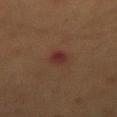Part of a total-body skin-imaging series; this lesion was reviewed on a skin check and was not flagged for biopsy. A 15 mm close-up extracted from a 3D total-body photography capture. The subject is a male in their mid-50s. Located on the abdomen.